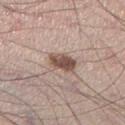Captured during whole-body skin photography for melanoma surveillance; the lesion was not biopsied.
Automated image analysis of the tile measured a lesion color around L≈51 a*≈18 b*≈24 in CIELAB and a lesion–skin lightness drop of about 14. The software also gave a border-irregularity index near 2/10, a within-lesion color-variation index near 4.5/10, and radial color variation of about 1.5. It also reported a classifier nevus-likeness of about 95/100 and lesion-presence confidence of about 100/100.
Measured at roughly 3.5 mm in maximum diameter.
A male subject aged around 35.
A roughly 15 mm field-of-view crop from a total-body skin photograph.
On the right lower leg.